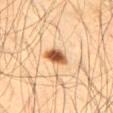biopsy status: no biopsy performed (imaged during a skin exam); body site: the right thigh; subject: male, aged 33 to 37; imaging modality: ~15 mm crop, total-body skin-cancer survey; lesion size: ~3 mm (longest diameter).